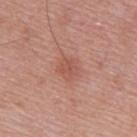Case summary:
- follow-up · imaged on a skin check; not biopsied
- body site · the upper back
- TBP lesion metrics · a footprint of about 4.5 mm², an eccentricity of roughly 0.4, and two-axis asymmetry of about 0.35; a lesion color around L≈54 a*≈25 b*≈28 in CIELAB and a normalized lesion–skin contrast near 5; a border-irregularity index near 3/10
- size · about 2.5 mm
- subject · male, roughly 50 years of age
- image · total-body-photography crop, ~15 mm field of view